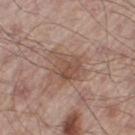Impression: The lesion was photographed on a routine skin check and not biopsied; there is no pathology result. Background: Located on the right thigh. A male patient about 55 years old. Measured at roughly 4 mm in maximum diameter. Imaged with white-light lighting. A lesion tile, about 15 mm wide, cut from a 3D total-body photograph.